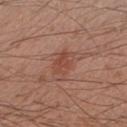anatomic site: the right forearm
imaging modality: 15 mm crop, total-body photography
subject: male, in their 30s